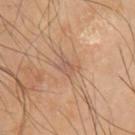<case>
  <biopsy_status>not biopsied; imaged during a skin examination</biopsy_status>
  <site>arm</site>
  <lighting>cross-polarized</lighting>
  <patient>
    <sex>male</sex>
    <age_approx>65</age_approx>
  </patient>
  <lesion_size>
    <long_diameter_mm_approx>2.5</long_diameter_mm_approx>
  </lesion_size>
  <image>
    <source>total-body photography crop</source>
    <field_of_view_mm>15</field_of_view_mm>
  </image>
</case>